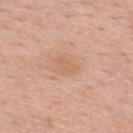Imaged during a routine full-body skin examination; the lesion was not biopsied and no histopathology is available. A 15 mm close-up extracted from a 3D total-body photography capture. Automated tile analysis of the lesion measured an outline eccentricity of about 0.7 (0 = round, 1 = elongated). And it measured a border-irregularity rating of about 3/10 and a within-lesion color-variation index near 1.5/10. And it measured a classifier nevus-likeness of about 0/100 and lesion-presence confidence of about 100/100. The lesion is located on the upper back. The tile uses white-light illumination. A male subject in their mid-60s.